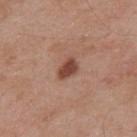Part of a total-body skin-imaging series; this lesion was reviewed on a skin check and was not flagged for biopsy.
Located on the upper back.
Approximately 3 mm at its widest.
A roughly 15 mm field-of-view crop from a total-body skin photograph.
A male subject aged around 55.
The tile uses white-light illumination.